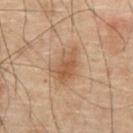{"site": "abdomen", "lesion_size": {"long_diameter_mm_approx": 3.5}, "lighting": "cross-polarized", "image": {"source": "total-body photography crop", "field_of_view_mm": 15}, "patient": {"sex": "male", "age_approx": 70}}